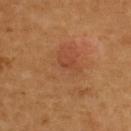  patient:
    sex: female
    age_approx: 60
  site: upper back
  lighting: cross-polarized
  image:
    source: total-body photography crop
    field_of_view_mm: 15